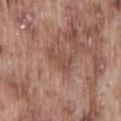Part of a total-body skin-imaging series; this lesion was reviewed on a skin check and was not flagged for biopsy. This image is a 15 mm lesion crop taken from a total-body photograph. This is a white-light tile. The lesion's longest dimension is about 3.5 mm. The patient is a male approximately 75 years of age. The lesion is located on the lower back.